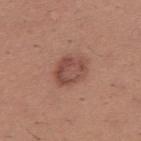Assessment: Imaged during a routine full-body skin examination; the lesion was not biopsied and no histopathology is available. Acquisition and patient details: From the right upper arm. Automated image analysis of the tile measured an area of roughly 10 mm² and a symmetry-axis asymmetry near 0.15. And it measured a border-irregularity index near 2/10, a within-lesion color-variation index near 4.5/10, and radial color variation of about 1.5. The software also gave a nevus-likeness score of about 60/100 and a detector confidence of about 100 out of 100 that the crop contains a lesion. A male patient, approximately 35 years of age. This is a white-light tile. Approximately 4 mm at its widest. This image is a 15 mm lesion crop taken from a total-body photograph.